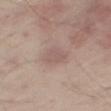<record>
  <biopsy_status>not biopsied; imaged during a skin examination</biopsy_status>
  <site>right thigh</site>
  <patient>
    <sex>male</sex>
    <age_approx>65</age_approx>
  </patient>
  <image>
    <source>total-body photography crop</source>
    <field_of_view_mm>15</field_of_view_mm>
  </image>
  <lighting>white-light</lighting>
  <lesion_size>
    <long_diameter_mm_approx>3.0</long_diameter_mm_approx>
  </lesion_size>
</record>